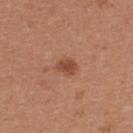Case summary:
• follow-up: no biopsy performed (imaged during a skin exam)
• automated lesion analysis: an area of roughly 4.5 mm², a shape eccentricity near 0.6, and a shape-asymmetry score of about 0.25 (0 = symmetric); internal color variation of about 2 on a 0–10 scale and radial color variation of about 0.5
• imaging modality: total-body-photography crop, ~15 mm field of view
• anatomic site: the upper back
• subject: female, aged 23–27
• lighting: white-light illumination
• size: ≈2.5 mm